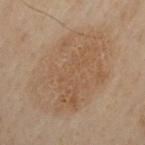Impression: No biopsy was performed on this lesion — it was imaged during a full skin examination and was not determined to be concerning. Image and clinical context: On the left upper arm. The tile uses cross-polarized illumination. A male subject, roughly 50 years of age. A roughly 15 mm field-of-view crop from a total-body skin photograph. About 11 mm across.